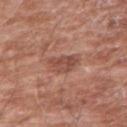Assessment: Captured during whole-body skin photography for melanoma surveillance; the lesion was not biopsied. Image and clinical context: A male patient, aged 58 to 62. The recorded lesion diameter is about 3.5 mm. A close-up tile cropped from a whole-body skin photograph, about 15 mm across. On the right upper arm. Captured under white-light illumination.